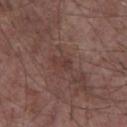Part of a total-body skin-imaging series; this lesion was reviewed on a skin check and was not flagged for biopsy. On the chest. The total-body-photography lesion software estimated a lesion area of about 2.5 mm², a shape eccentricity near 0.9, and a shape-asymmetry score of about 0.4 (0 = symmetric). The software also gave a mean CIELAB color near L≈37 a*≈19 b*≈21, about 6 CIELAB-L* units darker than the surrounding skin, and a normalized lesion–skin contrast near 5.5. A 15 mm crop from a total-body photograph taken for skin-cancer surveillance. The tile uses white-light illumination. A male patient in their 60s. Longest diameter approximately 2.5 mm.